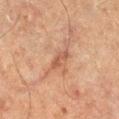Captured during whole-body skin photography for melanoma surveillance; the lesion was not biopsied. Longest diameter approximately 3 mm. The tile uses cross-polarized illumination. The lesion is located on the right lower leg. A female patient aged approximately 60. A 15 mm close-up extracted from a 3D total-body photography capture.